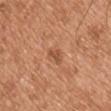Findings:
– notes — imaged on a skin check; not biopsied
– subject — male, aged approximately 55
– image — ~15 mm crop, total-body skin-cancer survey
– TBP lesion metrics — a lesion area of about 2.5 mm², an eccentricity of roughly 0.85, and a symmetry-axis asymmetry near 0.4; a border-irregularity rating of about 4/10 and a within-lesion color-variation index near 0.5/10; lesion-presence confidence of about 100/100
– body site — the right upper arm
– tile lighting — white-light illumination